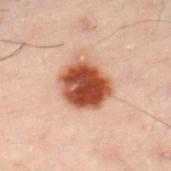This lesion was catalogued during total-body skin photography and was not selected for biopsy. Automated image analysis of the tile measured a mean CIELAB color near L≈41 a*≈25 b*≈29 and a lesion–skin lightness drop of about 18. The analysis additionally found a nevus-likeness score of about 100/100 and a lesion-detection confidence of about 100/100. A 15 mm crop from a total-body photograph taken for skin-cancer surveillance. Located on the left leg. A male patient, roughly 50 years of age. The recorded lesion diameter is about 4.5 mm.